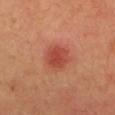Recorded during total-body skin imaging; not selected for excision or biopsy. The lesion's longest dimension is about 3 mm. The lesion is located on the head or neck. A 15 mm crop from a total-body photograph taken for skin-cancer surveillance. The lesion-visualizer software estimated a footprint of about 8 mm² and a shape eccentricity near 0.3. It also reported a border-irregularity rating of about 1.5/10, a within-lesion color-variation index near 2.5/10, and a peripheral color-asymmetry measure near 0.5. A female subject in their 40s.